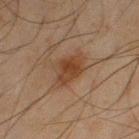Assessment:
Part of a total-body skin-imaging series; this lesion was reviewed on a skin check and was not flagged for biopsy.
Background:
The lesion is located on the left thigh. A male patient aged around 45. Imaged with cross-polarized lighting. A region of skin cropped from a whole-body photographic capture, roughly 15 mm wide. Automated image analysis of the tile measured a mean CIELAB color near L≈33 a*≈17 b*≈27, roughly 8 lightness units darker than nearby skin, and a lesion-to-skin contrast of about 8 (normalized; higher = more distinct). Longest diameter approximately 4.5 mm.